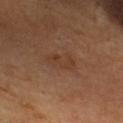workup = imaged on a skin check; not biopsied | lesion diameter = ~3.5 mm (longest diameter) | lighting = cross-polarized | imaging modality = ~15 mm tile from a whole-body skin photo | patient = female, in their 70s | body site = the head or neck | automated metrics = a classifier nevus-likeness of about 0/100 and a lesion-detection confidence of about 100/100.